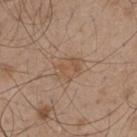biopsy_status: not biopsied; imaged during a skin examination
image:
  source: total-body photography crop
  field_of_view_mm: 15
patient:
  sex: male
  age_approx: 50
site: back